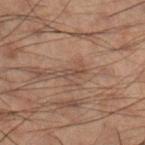No biopsy was performed on this lesion — it was imaged during a full skin examination and was not determined to be concerning. Automated tile analysis of the lesion measured a lesion area of about 3 mm², a shape eccentricity near 0.95, and two-axis asymmetry of about 0.4. And it measured a within-lesion color-variation index near 0/10 and a peripheral color-asymmetry measure near 0. It also reported a lesion-detection confidence of about 75/100. Longest diameter approximately 3.5 mm. The lesion is located on the right lower leg. A male subject, roughly 50 years of age. Cropped from a total-body skin-imaging series; the visible field is about 15 mm. Imaged with cross-polarized lighting.